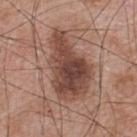biopsy_status: not biopsied; imaged during a skin examination
lesion_size:
  long_diameter_mm_approx: 8.0
automated_metrics:
  shape_asymmetry: 0.35
  nevus_likeness_0_100: 10
  lesion_detection_confidence_0_100: 100
lighting: white-light
patient:
  sex: male
  age_approx: 65
site: upper back
image:
  source: total-body photography crop
  field_of_view_mm: 15